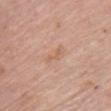This lesion was catalogued during total-body skin photography and was not selected for biopsy. On the chest. A close-up tile cropped from a whole-body skin photograph, about 15 mm across. This is a white-light tile. The patient is a female approximately 65 years of age. Measured at roughly 3 mm in maximum diameter.